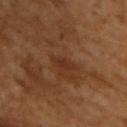{
  "biopsy_status": "not biopsied; imaged during a skin examination",
  "image": {
    "source": "total-body photography crop",
    "field_of_view_mm": 15
  },
  "patient": {
    "sex": "male",
    "age_approx": 65
  }
}